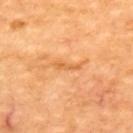{
  "lesion_size": {
    "long_diameter_mm_approx": 3.0
  },
  "patient": {
    "age_approx": 65
  },
  "image": {
    "source": "total-body photography crop",
    "field_of_view_mm": 15
  },
  "site": "upper back"
}